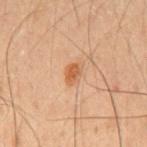The lesion was tiled from a total-body skin photograph and was not biopsied.
A male patient aged 48 to 52.
Located on the chest.
A 15 mm close-up extracted from a 3D total-body photography capture.
Longest diameter approximately 2.5 mm.